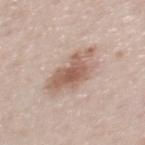The lesion was photographed on a routine skin check and not biopsied; there is no pathology result. The lesion is on the upper back. A close-up tile cropped from a whole-body skin photograph, about 15 mm across. A male subject, aged around 40. About 5.5 mm across.